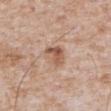biopsy status: no biopsy performed (imaged during a skin exam)
subject: male, aged 63–67
imaging modality: 15 mm crop, total-body photography
anatomic site: the abdomen